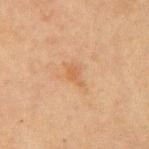{"biopsy_status": "not biopsied; imaged during a skin examination", "image": {"source": "total-body photography crop", "field_of_view_mm": 15}, "patient": {"sex": "male", "age_approx": 65}, "site": "left upper arm"}